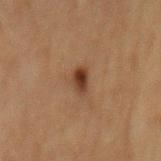Case summary:
- notes — no biopsy performed (imaged during a skin exam)
- lesion diameter — ≈3 mm
- tile lighting — cross-polarized illumination
- body site — the mid back
- patient — female, roughly 60 years of age
- acquisition — total-body-photography crop, ~15 mm field of view